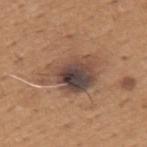<lesion>
<biopsy_status>not biopsied; imaged during a skin examination</biopsy_status>
<lesion_size>
  <long_diameter_mm_approx>6.5</long_diameter_mm_approx>
</lesion_size>
<image>
  <source>total-body photography crop</source>
  <field_of_view_mm>15</field_of_view_mm>
</image>
<site>upper back</site>
<patient>
  <sex>male</sex>
  <age_approx>65</age_approx>
</patient>
<automated_metrics>
  <border_irregularity_0_10>4.5</border_irregularity_0_10>
  <color_variation_0_10>9.0</color_variation_0_10>
  <peripheral_color_asymmetry>2.5</peripheral_color_asymmetry>
  <nevus_likeness_0_100>55</nevus_likeness_0_100>
  <lesion_detection_confidence_0_100>100</lesion_detection_confidence_0_100>
</automated_metrics>
<lighting>white-light</lighting>
</lesion>